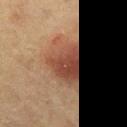Part of a total-body skin-imaging series; this lesion was reviewed on a skin check and was not flagged for biopsy.
From the left thigh.
About 6.5 mm across.
A region of skin cropped from a whole-body photographic capture, roughly 15 mm wide.
The patient is a female aged 48 to 52.